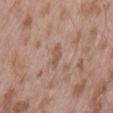workup: imaged on a skin check; not biopsied
patient: male, in their mid- to late 50s
lesion diameter: ≈3 mm
lighting: white-light illumination
anatomic site: the lower back
image: ~15 mm crop, total-body skin-cancer survey